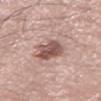| key | value |
|---|---|
| notes | total-body-photography surveillance lesion; no biopsy |
| lesion size | about 3.5 mm |
| lighting | white-light illumination |
| imaging modality | ~15 mm tile from a whole-body skin photo |
| patient | male, in their 70s |
| anatomic site | the left thigh |